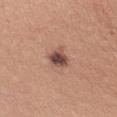No biopsy was performed on this lesion — it was imaged during a full skin examination and was not determined to be concerning.